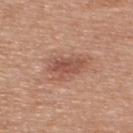The lesion was tiled from a total-body skin photograph and was not biopsied.
Measured at roughly 4.5 mm in maximum diameter.
The tile uses white-light illumination.
This image is a 15 mm lesion crop taken from a total-body photograph.
A male subject, in their 50s.
Automated image analysis of the tile measured a footprint of about 9 mm², a shape eccentricity near 0.75, and two-axis asymmetry of about 0.3. The software also gave an average lesion color of about L≈52 a*≈23 b*≈28 (CIELAB), about 10 CIELAB-L* units darker than the surrounding skin, and a normalized lesion–skin contrast near 7. It also reported a border-irregularity rating of about 3.5/10, internal color variation of about 3.5 on a 0–10 scale, and peripheral color asymmetry of about 1. It also reported a nevus-likeness score of about 40/100 and a detector confidence of about 100 out of 100 that the crop contains a lesion.
From the upper back.